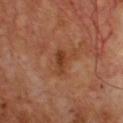{
  "automated_metrics": {
    "vs_skin_darker_L": 9.0,
    "vs_skin_contrast_norm": 7.5,
    "border_irregularity_0_10": 2.5,
    "color_variation_0_10": 1.0,
    "peripheral_color_asymmetry": 0.5
  },
  "lesion_size": {
    "long_diameter_mm_approx": 3.0
  },
  "patient": {
    "sex": "male",
    "age_approx": 65
  },
  "lighting": "cross-polarized",
  "image": {
    "source": "total-body photography crop",
    "field_of_view_mm": 15
  }
}